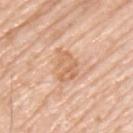subject — male, roughly 80 years of age; tile lighting — white-light illumination; lesion size — about 3.5 mm; image source — 15 mm crop, total-body photography; location — the upper back.